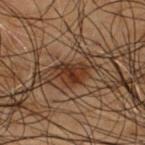notes — catalogued during a skin exam; not biopsied
image-analysis metrics — a lesion color around L≈23 a*≈16 b*≈23 in CIELAB and roughly 8 lightness units darker than nearby skin; a border-irregularity index near 4/10 and peripheral color asymmetry of about 1
image source — ~15 mm crop, total-body skin-cancer survey
anatomic site — the upper back
tile lighting — cross-polarized
diameter — ≈3 mm
subject — male, aged 48–52